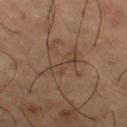| field | value |
|---|---|
| follow-up | no biopsy performed (imaged during a skin exam) |
| image source | ~15 mm tile from a whole-body skin photo |
| diameter | about 5 mm |
| subject | male, about 65 years old |
| tile lighting | cross-polarized illumination |
| anatomic site | the right thigh |